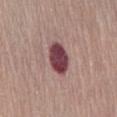Imaged during a routine full-body skin examination; the lesion was not biopsied and no histopathology is available.
Measured at roughly 4 mm in maximum diameter.
Imaged with white-light lighting.
The lesion is located on the abdomen.
A region of skin cropped from a whole-body photographic capture, roughly 15 mm wide.
Automated tile analysis of the lesion measured a lesion area of about 9 mm², an outline eccentricity of about 0.75 (0 = round, 1 = elongated), and a symmetry-axis asymmetry near 0.15. The analysis additionally found a within-lesion color-variation index near 4.5/10 and a peripheral color-asymmetry measure near 1.5.
A female patient, aged approximately 65.